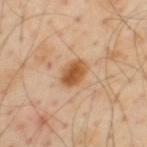<lesion>
<automated_metrics>
  <eccentricity>0.75</eccentricity>
  <shape_asymmetry>0.15</shape_asymmetry>
  <cielab_L>56</cielab_L>
  <cielab_a>22</cielab_a>
  <cielab_b>39</cielab_b>
  <vs_skin_contrast_norm>9.5</vs_skin_contrast_norm>
  <lesion_detection_confidence_0_100>100</lesion_detection_confidence_0_100>
</automated_metrics>
<lesion_size>
  <long_diameter_mm_approx>3.5</long_diameter_mm_approx>
</lesion_size>
<image>
  <source>total-body photography crop</source>
  <field_of_view_mm>15</field_of_view_mm>
</image>
<lighting>cross-polarized</lighting>
<site>upper back</site>
<patient>
  <sex>male</sex>
  <age_approx>55</age_approx>
</patient>
</lesion>